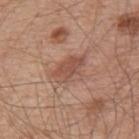Impression: The lesion was tiled from a total-body skin photograph and was not biopsied. Clinical summary: A close-up tile cropped from a whole-body skin photograph, about 15 mm across. On the upper back. A male patient in their mid-50s.